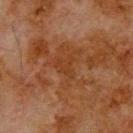{
  "biopsy_status": "not biopsied; imaged during a skin examination",
  "lighting": "cross-polarized",
  "automated_metrics": {
    "cielab_L": 30,
    "cielab_a": 20,
    "cielab_b": 29,
    "vs_skin_darker_L": 4.0,
    "vs_skin_contrast_norm": 4.5,
    "nevus_likeness_0_100": 0
  },
  "patient": {
    "sex": "male",
    "age_approx": 80
  },
  "image": {
    "source": "total-body photography crop",
    "field_of_view_mm": 15
  },
  "site": "upper back"
}